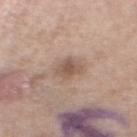Assessment: No biopsy was performed on this lesion — it was imaged during a full skin examination and was not determined to be concerning. Context: A female subject in their mid-50s. Captured under white-light illumination. About 3 mm across. A close-up tile cropped from a whole-body skin photograph, about 15 mm across. The lesion is on the left lower leg.